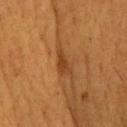Case summary:
• biopsy status: no biopsy performed (imaged during a skin exam)